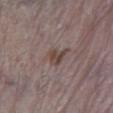- workup · imaged on a skin check; not biopsied
- image source · total-body-photography crop, ~15 mm field of view
- subject · male, approximately 70 years of age
- anatomic site · the right lower leg